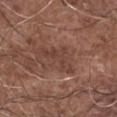Assessment:
This lesion was catalogued during total-body skin photography and was not selected for biopsy.
Clinical summary:
A male subject, in their mid- to late 70s. The lesion is on the right forearm. A region of skin cropped from a whole-body photographic capture, roughly 15 mm wide. The tile uses white-light illumination. Automated tile analysis of the lesion measured a symmetry-axis asymmetry near 0.75. The software also gave a mean CIELAB color near L≈40 a*≈20 b*≈24, a lesion–skin lightness drop of about 7, and a lesion-to-skin contrast of about 6 (normalized; higher = more distinct). The software also gave a border-irregularity rating of about 10/10, a within-lesion color-variation index near 1/10, and a peripheral color-asymmetry measure near 0. Longest diameter approximately 5 mm.